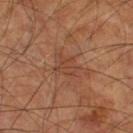workup=total-body-photography surveillance lesion; no biopsy
acquisition=~15 mm crop, total-body skin-cancer survey
site=the right thigh
patient=male, approximately 60 years of age
size=about 3 mm
illumination=cross-polarized illumination
automated metrics=border irregularity of about 4.5 on a 0–10 scale and internal color variation of about 1.5 on a 0–10 scale; a nevus-likeness score of about 0/100